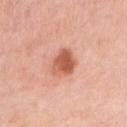Impression:
Recorded during total-body skin imaging; not selected for excision or biopsy.
Background:
From the right upper arm. This image is a 15 mm lesion crop taken from a total-body photograph. Imaged with white-light lighting. Approximately 3.5 mm at its widest. Automated image analysis of the tile measured a detector confidence of about 100 out of 100 that the crop contains a lesion. The patient is a female in their mid-60s.